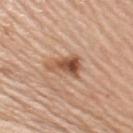Q: Is there a histopathology result?
A: imaged on a skin check; not biopsied
Q: How was the tile lit?
A: white-light illumination
Q: What is the lesion's diameter?
A: ~4.5 mm (longest diameter)
Q: What is the anatomic site?
A: the right upper arm
Q: How was this image acquired?
A: ~15 mm tile from a whole-body skin photo
Q: What did automated image analysis measure?
A: an area of roughly 7.5 mm² and a shape eccentricity near 0.9; a classifier nevus-likeness of about 95/100 and a detector confidence of about 100 out of 100 that the crop contains a lesion
Q: Who is the patient?
A: female, approximately 65 years of age